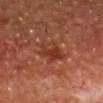{
  "biopsy_status": "not biopsied; imaged during a skin examination",
  "site": "head or neck",
  "patient": {
    "sex": "male",
    "age_approx": 65
  },
  "image": {
    "source": "total-body photography crop",
    "field_of_view_mm": 15
  }
}